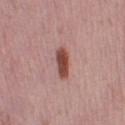This lesion was catalogued during total-body skin photography and was not selected for biopsy. Imaged with white-light lighting. The subject is a female in their 40s. A 15 mm close-up extracted from a 3D total-body photography capture. The lesion is located on the right thigh. The recorded lesion diameter is about 3.5 mm.